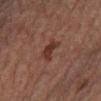Assessment: No biopsy was performed on this lesion — it was imaged during a full skin examination and was not determined to be concerning. Background: Imaged with cross-polarized lighting. Measured at roughly 3.5 mm in maximum diameter. Located on the right lower leg. A female patient roughly 55 years of age. The total-body-photography lesion software estimated a lesion area of about 4.5 mm², an eccentricity of roughly 0.85, and two-axis asymmetry of about 0.55. And it measured a border-irregularity index near 5.5/10. The analysis additionally found a classifier nevus-likeness of about 60/100 and a detector confidence of about 100 out of 100 that the crop contains a lesion. A lesion tile, about 15 mm wide, cut from a 3D total-body photograph.